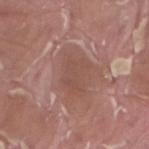Assessment:
The lesion was tiled from a total-body skin photograph and was not biopsied.
Image and clinical context:
About 4 mm across. The tile uses white-light illumination. A male patient in their 40s. Located on the right thigh. Cropped from a total-body skin-imaging series; the visible field is about 15 mm. The total-body-photography lesion software estimated a lesion area of about 10 mm² and an outline eccentricity of about 0.65 (0 = round, 1 = elongated). The software also gave a classifier nevus-likeness of about 0/100 and a lesion-detection confidence of about 85/100.